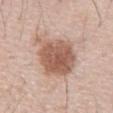<record>
<biopsy_status>not biopsied; imaged during a skin examination</biopsy_status>
<image>
  <source>total-body photography crop</source>
  <field_of_view_mm>15</field_of_view_mm>
</image>
<patient>
  <sex>male</sex>
  <age_approx>70</age_approx>
</patient>
<lighting>white-light</lighting>
<site>chest</site>
<automated_metrics>
  <vs_skin_darker_L>14.0</vs_skin_darker_L>
  <vs_skin_contrast_norm>9.0</vs_skin_contrast_norm>
  <lesion_detection_confidence_0_100>100</lesion_detection_confidence_0_100>
</automated_metrics>
</record>